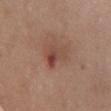workup: total-body-photography surveillance lesion; no biopsy | lesion size: ≈3.5 mm | location: the chest | illumination: white-light illumination | subject: female, aged around 65 | acquisition: ~15 mm tile from a whole-body skin photo.